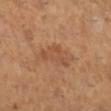patient: female, aged around 55; illumination: cross-polarized illumination; site: the right lower leg; lesion diameter: ~5 mm (longest diameter); imaging modality: ~15 mm crop, total-body skin-cancer survey.